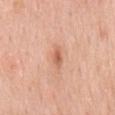follow-up: total-body-photography surveillance lesion; no biopsy | anatomic site: the mid back | lighting: white-light | subject: male, aged approximately 60 | acquisition: ~15 mm crop, total-body skin-cancer survey.